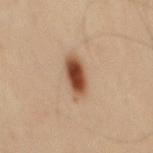Impression:
This lesion was catalogued during total-body skin photography and was not selected for biopsy.
Clinical summary:
A lesion tile, about 15 mm wide, cut from a 3D total-body photograph. A male patient, aged 43–47. Approximately 4.5 mm at its widest. Automated tile analysis of the lesion measured a footprint of about 7.5 mm², a shape eccentricity near 0.85, and a symmetry-axis asymmetry near 0.25. It also reported a lesion color around L≈48 a*≈22 b*≈32 in CIELAB, roughly 18 lightness units darker than nearby skin, and a lesion-to-skin contrast of about 12 (normalized; higher = more distinct). And it measured border irregularity of about 2.5 on a 0–10 scale. On the mid back.